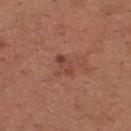Q: Is there a histopathology result?
A: total-body-photography surveillance lesion; no biopsy
Q: What kind of image is this?
A: ~15 mm crop, total-body skin-cancer survey
Q: Automated lesion metrics?
A: a lesion area of about 3 mm², a shape eccentricity near 0.9, and a shape-asymmetry score of about 0.2 (0 = symmetric); a border-irregularity index near 2.5/10 and radial color variation of about 0; a nevus-likeness score of about 0/100 and a lesion-detection confidence of about 100/100
Q: How large is the lesion?
A: about 3 mm
Q: Lesion location?
A: the upper back
Q: Patient demographics?
A: female, aged 28–32